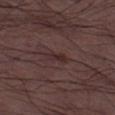Findings:
– follow-up · no biopsy performed (imaged during a skin exam)
– automated metrics · a lesion area of about 3 mm², an outline eccentricity of about 0.95 (0 = round, 1 = elongated), and a shape-asymmetry score of about 0.25 (0 = symmetric); border irregularity of about 3 on a 0–10 scale, a color-variation rating of about 0/10, and a peripheral color-asymmetry measure near 0; a nevus-likeness score of about 0/100 and lesion-presence confidence of about 75/100
– image source · ~15 mm crop, total-body skin-cancer survey
– anatomic site · the left thigh
– lesion diameter · about 3 mm
– subject · male, aged 48–52
– tile lighting · white-light illumination